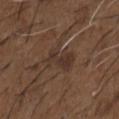  lighting: white-light
  site: chest
  lesion_size:
    long_diameter_mm_approx: 4.5
  image:
    source: total-body photography crop
    field_of_view_mm: 15
  patient:
    sex: male
    age_approx: 50
  automated_metrics:
    border_irregularity_0_10: 4.5
    color_variation_0_10: 3.0
    peripheral_color_asymmetry: 1.0